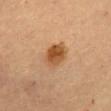Impression: No biopsy was performed on this lesion — it was imaged during a full skin examination and was not determined to be concerning. Context: The patient is a female about 60 years old. Longest diameter approximately 3.5 mm. Captured under cross-polarized illumination. Located on the chest. A 15 mm close-up tile from a total-body photography series done for melanoma screening.